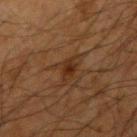Impression:
This lesion was catalogued during total-body skin photography and was not selected for biopsy.
Acquisition and patient details:
The patient is a male aged 63–67. A 15 mm close-up tile from a total-body photography series done for melanoma screening. From the right upper arm.